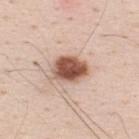The lesion was tiled from a total-body skin photograph and was not biopsied. Approximately 4.5 mm at its widest. The patient is a male aged around 35. Located on the upper back. The total-body-photography lesion software estimated a lesion color around L≈56 a*≈21 b*≈28 in CIELAB and roughly 19 lightness units darker than nearby skin. The software also gave a within-lesion color-variation index near 6/10 and peripheral color asymmetry of about 2. Cropped from a total-body skin-imaging series; the visible field is about 15 mm.